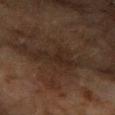follow-up: catalogued during a skin exam; not biopsied
imaging modality: ~15 mm tile from a whole-body skin photo
automated lesion analysis: a lesion color around L≈21 a*≈12 b*≈20 in CIELAB and roughly 5 lightness units darker than nearby skin
subject: female, roughly 60 years of age
body site: the left forearm
tile lighting: cross-polarized illumination
lesion size: about 5 mm